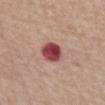<lesion>
<biopsy_status>not biopsied; imaged during a skin examination</biopsy_status>
<site>abdomen</site>
<image>
  <source>total-body photography crop</source>
  <field_of_view_mm>15</field_of_view_mm>
</image>
<lesion_size>
  <long_diameter_mm_approx>3.0</long_diameter_mm_approx>
</lesion_size>
<patient>
  <sex>male</sex>
  <age_approx>75</age_approx>
</patient>
<automated_metrics>
  <area_mm2_approx>8.0</area_mm2_approx>
  <eccentricity>0.35</eccentricity>
  <shape_asymmetry>0.15</shape_asymmetry>
</automated_metrics>
<lighting>white-light</lighting>
</lesion>